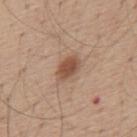Notes:
• workup — total-body-photography surveillance lesion; no biopsy
• anatomic site — the mid back
• subject — male, aged approximately 55
• acquisition — 15 mm crop, total-body photography
• diameter — ≈3 mm